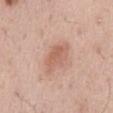Impression: Part of a total-body skin-imaging series; this lesion was reviewed on a skin check and was not flagged for biopsy. Context: A male patient aged around 55. A roughly 15 mm field-of-view crop from a total-body skin photograph. An algorithmic analysis of the crop reported a lesion area of about 7 mm² and two-axis asymmetry of about 0.2. It also reported internal color variation of about 2.5 on a 0–10 scale. And it measured a classifier nevus-likeness of about 35/100 and a detector confidence of about 100 out of 100 that the crop contains a lesion. Located on the abdomen.